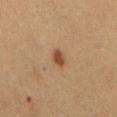{"biopsy_status": "not biopsied; imaged during a skin examination", "lighting": "cross-polarized", "site": "mid back", "image": {"source": "total-body photography crop", "field_of_view_mm": 15}, "patient": {"sex": "female", "age_approx": 40}}